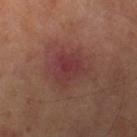Imaged during a routine full-body skin examination; the lesion was not biopsied and no histopathology is available. A 15 mm close-up extracted from a 3D total-body photography capture. The lesion is on the leg. A male patient aged around 65. Imaged with cross-polarized lighting. Approximately 3.5 mm at its widest.